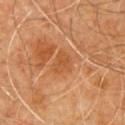Assessment:
Recorded during total-body skin imaging; not selected for excision or biopsy.
Background:
About 2.5 mm across. Located on the chest. A male patient about 60 years old. Cropped from a whole-body photographic skin survey; the tile spans about 15 mm.